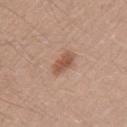Clinical summary: This is a white-light tile. Automated image analysis of the tile measured a color-variation rating of about 2/10 and a peripheral color-asymmetry measure near 0.5. Longest diameter approximately 3 mm. Cropped from a whole-body photographic skin survey; the tile spans about 15 mm. Located on the left upper arm. A male patient, in their 40s.